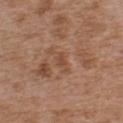No biopsy was performed on this lesion — it was imaged during a full skin examination and was not determined to be concerning.
The patient is a male aged 73–77.
The total-body-photography lesion software estimated a mean CIELAB color near L≈48 a*≈20 b*≈31, about 7 CIELAB-L* units darker than the surrounding skin, and a normalized lesion–skin contrast near 5.5.
Located on the chest.
A region of skin cropped from a whole-body photographic capture, roughly 15 mm wide.
Imaged with white-light lighting.
About 2.5 mm across.